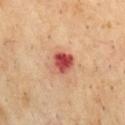Impression: This lesion was catalogued during total-body skin photography and was not selected for biopsy. Context: The subject is a male aged 68–72. Imaged with cross-polarized lighting. The recorded lesion diameter is about 3 mm. Automated tile analysis of the lesion measured a border-irregularity index near 2/10. And it measured a nevus-likeness score of about 0/100 and lesion-presence confidence of about 100/100. Located on the chest. This image is a 15 mm lesion crop taken from a total-body photograph.